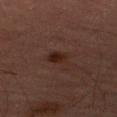Q: Is there a histopathology result?
A: catalogued during a skin exam; not biopsied